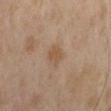notes = no biopsy performed (imaged during a skin exam)
site = the back
subject = male, aged 58–62
diameter = ~2.5 mm (longest diameter)
image-analysis metrics = a mean CIELAB color near L≈47 a*≈15 b*≈29, a lesion–skin lightness drop of about 6, and a normalized border contrast of about 6
lighting = cross-polarized illumination
imaging modality = total-body-photography crop, ~15 mm field of view